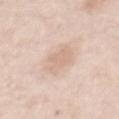{
  "biopsy_status": "not biopsied; imaged during a skin examination",
  "site": "abdomen",
  "lesion_size": {
    "long_diameter_mm_approx": 5.0
  },
  "patient": {
    "sex": "male",
    "age_approx": 50
  },
  "image": {
    "source": "total-body photography crop",
    "field_of_view_mm": 15
  },
  "automated_metrics": {
    "area_mm2_approx": 8.5,
    "shape_asymmetry": 0.2,
    "cielab_L": 71,
    "cielab_a": 16,
    "cielab_b": 28,
    "vs_skin_darker_L": 8.0,
    "vs_skin_contrast_norm": 5.0,
    "border_irregularity_0_10": 3.0,
    "color_variation_0_10": 1.5,
    "peripheral_color_asymmetry": 0.5
  },
  "lighting": "white-light"
}